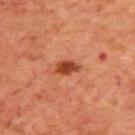* biopsy status — imaged on a skin check; not biopsied
* illumination — cross-polarized
* imaging modality — total-body-photography crop, ~15 mm field of view
* automated metrics — a footprint of about 4.5 mm², a shape eccentricity near 0.85, and a symmetry-axis asymmetry near 0.2; a lesion color around L≈44 a*≈30 b*≈37 in CIELAB, a lesion–skin lightness drop of about 14, and a lesion-to-skin contrast of about 10 (normalized; higher = more distinct); a border-irregularity index near 2.5/10 and radial color variation of about 0.5; an automated nevus-likeness rating near 95 out of 100
* anatomic site — the upper back
* lesion diameter — ≈3 mm
* subject — male, about 70 years old